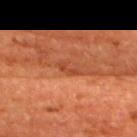No biopsy was performed on this lesion — it was imaged during a full skin examination and was not determined to be concerning.
A roughly 15 mm field-of-view crop from a total-body skin photograph.
The lesion is located on the back.
This is a cross-polarized tile.
The patient is a male aged 63 to 67.
Approximately 3 mm at its widest.